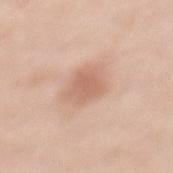notes — no biopsy performed (imaged during a skin exam)
acquisition — ~15 mm crop, total-body skin-cancer survey
site — the back
tile lighting — white-light illumination
subject — female, in their mid-30s